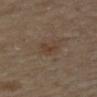This lesion was catalogued during total-body skin photography and was not selected for biopsy. A female subject approximately 60 years of age. From the upper back. A close-up tile cropped from a whole-body skin photograph, about 15 mm across.